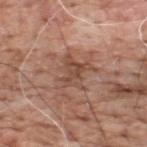Q: Is there a histopathology result?
A: total-body-photography surveillance lesion; no biopsy
Q: How large is the lesion?
A: ~4.5 mm (longest diameter)
Q: Illumination type?
A: white-light illumination
Q: Patient demographics?
A: male, approximately 60 years of age
Q: Lesion location?
A: the upper back
Q: What kind of image is this?
A: total-body-photography crop, ~15 mm field of view
Q: Automated lesion metrics?
A: an area of roughly 9.5 mm², an eccentricity of roughly 0.5, and a symmetry-axis asymmetry near 0.4; a mean CIELAB color near L≈49 a*≈20 b*≈28, a lesion–skin lightness drop of about 9, and a normalized border contrast of about 6.5; border irregularity of about 7 on a 0–10 scale, a color-variation rating of about 3/10, and radial color variation of about 1